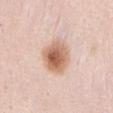notes: imaged on a skin check; not biopsied | illumination: white-light | location: the front of the torso | diameter: about 4.5 mm | image source: 15 mm crop, total-body photography | patient: female, aged approximately 40.